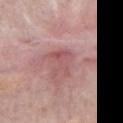Assessment: Imaged during a routine full-body skin examination; the lesion was not biopsied and no histopathology is available. Background: Approximately 3.5 mm at its widest. A female subject, aged around 70. Automated image analysis of the tile measured a lesion area of about 6 mm², a shape eccentricity near 0.65, and a symmetry-axis asymmetry near 0.6. The software also gave a mean CIELAB color near L≈54 a*≈25 b*≈21, roughly 7 lightness units darker than nearby skin, and a normalized border contrast of about 5. And it measured a nevus-likeness score of about 0/100. A close-up tile cropped from a whole-body skin photograph, about 15 mm across. Captured under white-light illumination. From the chest.